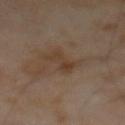{"biopsy_status": "not biopsied; imaged during a skin examination", "lighting": "cross-polarized", "patient": {"sex": "male", "age_approx": 65}, "lesion_size": {"long_diameter_mm_approx": 3.5}, "image": {"source": "total-body photography crop", "field_of_view_mm": 15}}